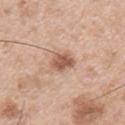Notes:
* image · total-body-photography crop, ~15 mm field of view
* anatomic site · the left upper arm
* patient · male, approximately 55 years of age